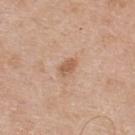Q: What is the lesion's diameter?
A: ~2.5 mm (longest diameter)
Q: Automated lesion metrics?
A: a shape-asymmetry score of about 0.25 (0 = symmetric); a color-variation rating of about 2/10 and a peripheral color-asymmetry measure near 0.5; a detector confidence of about 100 out of 100 that the crop contains a lesion
Q: Illumination type?
A: white-light
Q: Where on the body is the lesion?
A: the back
Q: What are the patient's age and sex?
A: male, about 55 years old
Q: What is the imaging modality?
A: ~15 mm crop, total-body skin-cancer survey